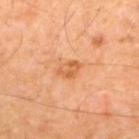workup — imaged on a skin check; not biopsied
subject — male, approximately 55 years of age
lighting — cross-polarized
imaging modality — total-body-photography crop, ~15 mm field of view
anatomic site — the upper back